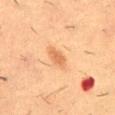This lesion was catalogued during total-body skin photography and was not selected for biopsy. The total-body-photography lesion software estimated border irregularity of about 2 on a 0–10 scale, a color-variation rating of about 1/10, and peripheral color asymmetry of about 0.5. Located on the back. The tile uses cross-polarized illumination. About 3 mm across. Cropped from a whole-body photographic skin survey; the tile spans about 15 mm. A male patient, aged 53–57.